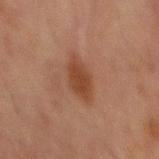Q: Was this lesion biopsied?
A: catalogued during a skin exam; not biopsied
Q: What are the patient's age and sex?
A: male, approximately 65 years of age
Q: How large is the lesion?
A: about 5 mm
Q: How was this image acquired?
A: ~15 mm tile from a whole-body skin photo
Q: Illumination type?
A: cross-polarized illumination
Q: Where on the body is the lesion?
A: the front of the torso